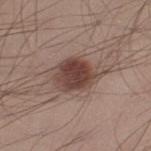Imaged during a routine full-body skin examination; the lesion was not biopsied and no histopathology is available. A male patient, roughly 30 years of age. The lesion is on the left thigh. Automated tile analysis of the lesion measured an area of roughly 16 mm², an eccentricity of roughly 0.75, and two-axis asymmetry of about 0.15. It also reported a lesion color around L≈44 a*≈18 b*≈22 in CIELAB, a lesion–skin lightness drop of about 13, and a normalized border contrast of about 10. And it measured a classifier nevus-likeness of about 90/100. A 15 mm close-up tile from a total-body photography series done for melanoma screening. This is a white-light tile.